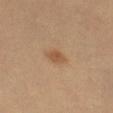Findings:
• notes — no biopsy performed (imaged during a skin exam)
• illumination — cross-polarized
• imaging modality — ~15 mm tile from a whole-body skin photo
• subject — aged 58 to 62
• size — ~2.5 mm (longest diameter)
• anatomic site — the leg